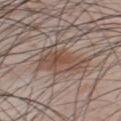Part of a total-body skin-imaging series; this lesion was reviewed on a skin check and was not flagged for biopsy. The lesion's longest dimension is about 5 mm. The lesion-visualizer software estimated two-axis asymmetry of about 0.35. A male subject, aged around 35. A 15 mm close-up tile from a total-body photography series done for melanoma screening. The tile uses white-light illumination. On the upper back.